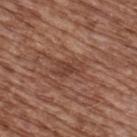follow-up: total-body-photography surveillance lesion; no biopsy | automated metrics: an area of roughly 5 mm², an outline eccentricity of about 0.85 (0 = round, 1 = elongated), and a shape-asymmetry score of about 0.3 (0 = symmetric); an average lesion color of about L≈41 a*≈22 b*≈27 (CIELAB), roughly 8 lightness units darker than nearby skin, and a lesion-to-skin contrast of about 6.5 (normalized; higher = more distinct); an automated nevus-likeness rating near 5 out of 100 and lesion-presence confidence of about 90/100 | subject: male, in their mid-70s | anatomic site: the back | image source: ~15 mm crop, total-body skin-cancer survey | lesion diameter: ~3.5 mm (longest diameter).